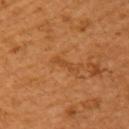Assessment: Part of a total-body skin-imaging series; this lesion was reviewed on a skin check and was not flagged for biopsy. Context: Cropped from a total-body skin-imaging series; the visible field is about 15 mm. Captured under cross-polarized illumination. A female subject aged 53–57. From the back. The recorded lesion diameter is about 2.5 mm.